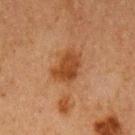follow-up: no biopsy performed (imaged during a skin exam) | body site: the left upper arm | diameter: ≈4 mm | lighting: cross-polarized | image-analysis metrics: a mean CIELAB color near L≈37 a*≈21 b*≈32, a lesion–skin lightness drop of about 9, and a normalized border contrast of about 8.5; a border-irregularity index near 2.5/10, internal color variation of about 2.5 on a 0–10 scale, and a peripheral color-asymmetry measure near 1; an automated nevus-likeness rating near 80 out of 100 and a lesion-detection confidence of about 100/100 | patient: female, aged around 60 | image source: 15 mm crop, total-body photography.